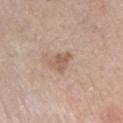The lesion was tiled from a total-body skin photograph and was not biopsied. The tile uses white-light illumination. On the left upper arm. A 15 mm crop from a total-body photograph taken for skin-cancer surveillance. A female subject roughly 65 years of age. The lesion's longest dimension is about 2.5 mm.